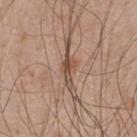Imaged during a routine full-body skin examination; the lesion was not biopsied and no histopathology is available.
An algorithmic analysis of the crop reported an average lesion color of about L≈48 a*≈19 b*≈28 (CIELAB), a lesion–skin lightness drop of about 12, and a normalized border contrast of about 9.
A 15 mm close-up extracted from a 3D total-body photography capture.
Captured under white-light illumination.
The subject is a male about 50 years old.
Located on the upper back.